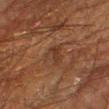follow-up — no biopsy performed (imaged during a skin exam) | subject — male, aged 63 to 67 | lighting — cross-polarized | diameter — about 3 mm | body site — the right lower leg | imaging modality — 15 mm crop, total-body photography | automated lesion analysis — an automated nevus-likeness rating near 0 out of 100 and lesion-presence confidence of about 95/100.